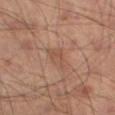No biopsy was performed on this lesion — it was imaged during a full skin examination and was not determined to be concerning.
A male patient, aged approximately 55.
This image is a 15 mm lesion crop taken from a total-body photograph.
Automated image analysis of the tile measured a footprint of about 4 mm², an outline eccentricity of about 0.8 (0 = round, 1 = elongated), and two-axis asymmetry of about 0.45. The software also gave roughly 6 lightness units darker than nearby skin and a lesion-to-skin contrast of about 5 (normalized; higher = more distinct). The analysis additionally found a color-variation rating of about 3/10.
Located on the left thigh.
This is a cross-polarized tile.